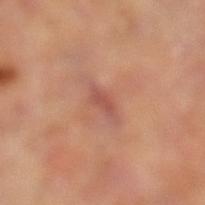follow-up = imaged on a skin check; not biopsied | site = the left thigh | acquisition = ~15 mm tile from a whole-body skin photo | size = ≈3 mm | illumination = cross-polarized illumination | automated metrics = a lesion–skin lightness drop of about 8 and a normalized lesion–skin contrast near 6; a border-irregularity index near 3.5/10, internal color variation of about 0 on a 0–10 scale, and peripheral color asymmetry of about 0.